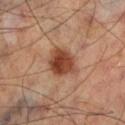Impression:
No biopsy was performed on this lesion — it was imaged during a full skin examination and was not determined to be concerning.
Image and clinical context:
The lesion-visualizer software estimated an average lesion color of about L≈45 a*≈24 b*≈31 (CIELAB), a lesion–skin lightness drop of about 14, and a lesion-to-skin contrast of about 10 (normalized; higher = more distinct). The analysis additionally found a color-variation rating of about 5/10 and a peripheral color-asymmetry measure near 1.5. The software also gave a lesion-detection confidence of about 100/100. The tile uses cross-polarized illumination. Located on the left lower leg. The patient is a male aged 53 to 57. A 15 mm close-up extracted from a 3D total-body photography capture.